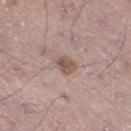| key | value |
|---|---|
| notes | no biopsy performed (imaged during a skin exam) |
| lesion diameter | ~2.5 mm (longest diameter) |
| subject | male, aged around 55 |
| lighting | white-light illumination |
| body site | the right thigh |
| imaging modality | ~15 mm tile from a whole-body skin photo |
| automated metrics | about 10 CIELAB-L* units darker than the surrounding skin and a normalized lesion–skin contrast near 7.5; border irregularity of about 2 on a 0–10 scale, internal color variation of about 3 on a 0–10 scale, and radial color variation of about 1 |